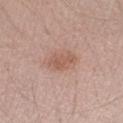Imaged during a routine full-body skin examination; the lesion was not biopsied and no histopathology is available.
The tile uses white-light illumination.
Automated image analysis of the tile measured an area of roughly 6.5 mm², a shape eccentricity near 0.8, and two-axis asymmetry of about 0.2. And it measured a border-irregularity index near 2.5/10, a within-lesion color-variation index near 2/10, and peripheral color asymmetry of about 0.5. The software also gave a classifier nevus-likeness of about 45/100 and a lesion-detection confidence of about 100/100.
Cropped from a whole-body photographic skin survey; the tile spans about 15 mm.
A male patient, aged 38–42.
The lesion is located on the left forearm.